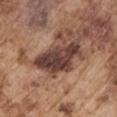Assessment: No biopsy was performed on this lesion — it was imaged during a full skin examination and was not determined to be concerning. Acquisition and patient details: Imaged with white-light lighting. Cropped from a whole-body photographic skin survey; the tile spans about 15 mm. Approximately 6.5 mm at its widest. The lesion is on the right upper arm. The subject is a male aged approximately 75.